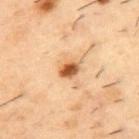biopsy_status: not biopsied; imaged during a skin examination
image:
  source: total-body photography crop
  field_of_view_mm: 15
site: back
patient:
  sex: male
  age_approx: 55
lighting: cross-polarized